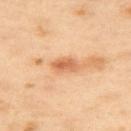  biopsy_status: not biopsied; imaged during a skin examination